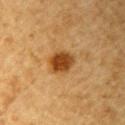Notes:
• biopsy status — catalogued during a skin exam; not biopsied
• size — ~3 mm (longest diameter)
• patient — male, aged 83 or older
• acquisition — ~15 mm tile from a whole-body skin photo
• lighting — cross-polarized illumination
• location — the right upper arm